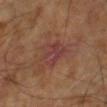Recorded during total-body skin imaging; not selected for excision or biopsy.
Located on the right arm.
The total-body-photography lesion software estimated a lesion area of about 9 mm², a shape eccentricity near 0.35, and two-axis asymmetry of about 0.3. It also reported a lesion color around L≈37 a*≈22 b*≈22 in CIELAB, roughly 5 lightness units darker than nearby skin, and a normalized border contrast of about 6.5. The software also gave a border-irregularity rating of about 3/10. The software also gave a nevus-likeness score of about 0/100 and lesion-presence confidence of about 100/100.
A male patient in their 60s.
Cropped from a whole-body photographic skin survey; the tile spans about 15 mm.